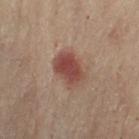Clinical impression:
Captured during whole-body skin photography for melanoma surveillance; the lesion was not biopsied.
Clinical summary:
The subject is a female approximately 60 years of age. Longest diameter approximately 4 mm. A 15 mm close-up tile from a total-body photography series done for melanoma screening. Located on the right thigh.